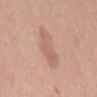notes = no biopsy performed (imaged during a skin exam); subject = female, in their 50s; lesion diameter = about 5 mm; body site = the back; image = ~15 mm crop, total-body skin-cancer survey; TBP lesion metrics = a border-irregularity rating of about 3/10, a color-variation rating of about 2.5/10, and a peripheral color-asymmetry measure near 1.